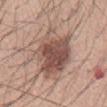A lesion tile, about 15 mm wide, cut from a 3D total-body photograph.
The lesion is on the mid back.
The tile uses white-light illumination.
Automated image analysis of the tile measured an area of roughly 26 mm² and an outline eccentricity of about 0.85 (0 = round, 1 = elongated). The software also gave about 14 CIELAB-L* units darker than the surrounding skin and a lesion-to-skin contrast of about 9.5 (normalized; higher = more distinct). The software also gave an automated nevus-likeness rating near 90 out of 100.
A male subject aged approximately 60.
The recorded lesion diameter is about 8.5 mm.